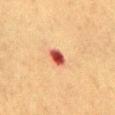| feature | finding |
|---|---|
| follow-up | catalogued during a skin exam; not biopsied |
| acquisition | total-body-photography crop, ~15 mm field of view |
| tile lighting | cross-polarized illumination |
| size | ≈2.5 mm |
| subject | female, in their mid-50s |
| image-analysis metrics | a border-irregularity rating of about 1.5/10 and a color-variation rating of about 4.5/10 |
| site | the front of the torso |